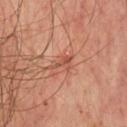Recorded during total-body skin imaging; not selected for excision or biopsy.